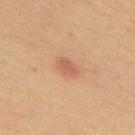notes: no biopsy performed (imaged during a skin exam)
subject: male, aged 58 to 62
acquisition: ~15 mm crop, total-body skin-cancer survey
illumination: cross-polarized
site: the upper back